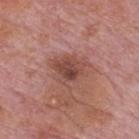{"biopsy_status": "not biopsied; imaged during a skin examination", "patient": {"sex": "male", "age_approx": 75}, "automated_metrics": {"area_mm2_approx": 7.5, "eccentricity": 0.5, "cielab_L": 45, "cielab_a": 23, "cielab_b": 25, "vs_skin_darker_L": 10.0, "vs_skin_contrast_norm": 7.5, "color_variation_0_10": 5.0, "peripheral_color_asymmetry": 2.0, "nevus_likeness_0_100": 0, "lesion_detection_confidence_0_100": 100}, "image": {"source": "total-body photography crop", "field_of_view_mm": 15}, "site": "mid back", "lesion_size": {"long_diameter_mm_approx": 3.5}}